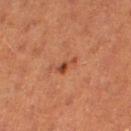Captured during whole-body skin photography for melanoma surveillance; the lesion was not biopsied.
A roughly 15 mm field-of-view crop from a total-body skin photograph.
About 2.5 mm across.
On the right thigh.
The subject is a female in their 40s.
This is a cross-polarized tile.